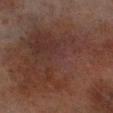Impression: The lesion was tiled from a total-body skin photograph and was not biopsied. Clinical summary: Located on the left lower leg. A male subject, aged approximately 70. Cropped from a total-body skin-imaging series; the visible field is about 15 mm. Measured at roughly 16 mm in maximum diameter. The tile uses cross-polarized illumination.